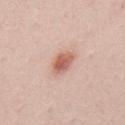{
  "biopsy_status": "not biopsied; imaged during a skin examination"
}